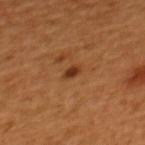No biopsy was performed on this lesion — it was imaged during a full skin examination and was not determined to be concerning. The subject is a male aged 43–47. This image is a 15 mm lesion crop taken from a total-body photograph. The lesion is on the upper back. About 2 mm across.